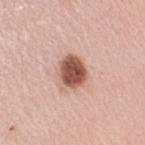{
  "biopsy_status": "not biopsied; imaged during a skin examination",
  "image": {
    "source": "total-body photography crop",
    "field_of_view_mm": 15
  },
  "site": "right upper arm",
  "patient": {
    "sex": "female",
    "age_approx": 50
  }
}